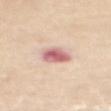notes = no biopsy performed (imaged during a skin exam); subject = female, in their mid-60s; imaging modality = ~15 mm crop, total-body skin-cancer survey; location = the mid back.